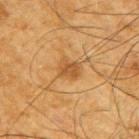<tbp_lesion>
  <biopsy_status>not biopsied; imaged during a skin examination</biopsy_status>
  <site>right upper arm</site>
  <lighting>cross-polarized</lighting>
  <automated_metrics>
    <shape_asymmetry>0.15</shape_asymmetry>
    <cielab_L>43</cielab_L>
    <cielab_a>19</cielab_a>
    <cielab_b>37</cielab_b>
    <vs_skin_darker_L>8.0</vs_skin_darker_L>
  </automated_metrics>
  <patient>
    <sex>male</sex>
    <age_approx>65</age_approx>
  </patient>
  <image>
    <source>total-body photography crop</source>
    <field_of_view_mm>15</field_of_view_mm>
  </image>
  <lesion_size>
    <long_diameter_mm_approx>2.5</long_diameter_mm_approx>
  </lesion_size>
</tbp_lesion>